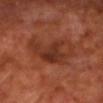notes = imaged on a skin check; not biopsied | imaging modality = ~15 mm tile from a whole-body skin photo | anatomic site = the chest | lighting = cross-polarized illumination | subject = male, aged approximately 70 | diameter = ≈6.5 mm.